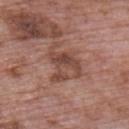follow-up: catalogued during a skin exam; not biopsied | imaging modality: 15 mm crop, total-body photography | site: the back | subject: male, aged 68–72.